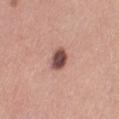Notes:
• lighting — white-light illumination
• acquisition — total-body-photography crop, ~15 mm field of view
• subject — female, aged 28–32
• site — the left thigh
• size — ~3 mm (longest diameter)